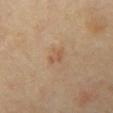Recorded during total-body skin imaging; not selected for excision or biopsy. Automated tile analysis of the lesion measured a footprint of about 4 mm², an eccentricity of roughly 0.8, and two-axis asymmetry of about 0.25. A male patient, aged around 60. On the chest. Captured under cross-polarized illumination. The recorded lesion diameter is about 3 mm. A roughly 15 mm field-of-view crop from a total-body skin photograph.